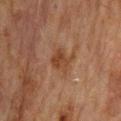notes: catalogued during a skin exam; not biopsied | tile lighting: cross-polarized | diameter: about 3 mm | patient: male, approximately 70 years of age | image-analysis metrics: a lesion color around L≈35 a*≈18 b*≈28 in CIELAB, a lesion–skin lightness drop of about 7, and a normalized lesion–skin contrast near 7; a border-irregularity index near 3/10, a within-lesion color-variation index near 3/10, and radial color variation of about 1; an automated nevus-likeness rating near 30 out of 100 and lesion-presence confidence of about 100/100 | imaging modality: 15 mm crop, total-body photography | site: the mid back.